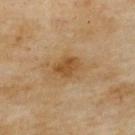Findings:
– follow-up — total-body-photography surveillance lesion; no biopsy
– location — the upper back
– lesion diameter — about 3 mm
– automated lesion analysis — an eccentricity of roughly 0.7 and a symmetry-axis asymmetry near 0.2; a normalized lesion–skin contrast near 8; a within-lesion color-variation index near 2.5/10 and radial color variation of about 1
– subject — female, aged 58 to 62
– lighting — cross-polarized
– image source — total-body-photography crop, ~15 mm field of view